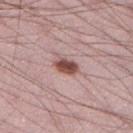notes: imaged on a skin check; not biopsied | image-analysis metrics: a footprint of about 5 mm² and an eccentricity of roughly 0.8; border irregularity of about 2 on a 0–10 scale and peripheral color asymmetry of about 1 | image: 15 mm crop, total-body photography | body site: the leg | lesion diameter: ≈3 mm | tile lighting: white-light | subject: male, approximately 25 years of age.